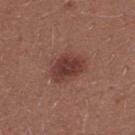Clinical impression: Part of a total-body skin-imaging series; this lesion was reviewed on a skin check and was not flagged for biopsy. Clinical summary: The total-body-photography lesion software estimated an eccentricity of roughly 0.75 and two-axis asymmetry of about 0.2. The patient is a female in their mid- to late 20s. The lesion is located on the upper back. Imaged with white-light lighting. A close-up tile cropped from a whole-body skin photograph, about 15 mm across. Approximately 4 mm at its widest.